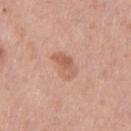Recorded during total-body skin imaging; not selected for excision or biopsy.
Captured under white-light illumination.
The subject is a female aged 53 to 57.
Automated tile analysis of the lesion measured roughly 8 lightness units darker than nearby skin and a lesion-to-skin contrast of about 6 (normalized; higher = more distinct). The software also gave a color-variation rating of about 4/10.
The lesion is on the left thigh.
Cropped from a whole-body photographic skin survey; the tile spans about 15 mm.
The recorded lesion diameter is about 4 mm.